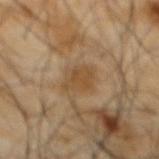<case>
<lighting>cross-polarized</lighting>
<image>
  <source>total-body photography crop</source>
  <field_of_view_mm>15</field_of_view_mm>
</image>
<patient>
  <sex>male</sex>
  <age_approx>65</age_approx>
</patient>
<site>mid back</site>
<automated_metrics>
  <nevus_likeness_0_100>25</nevus_likeness_0_100>
  <lesion_detection_confidence_0_100>100</lesion_detection_confidence_0_100>
</automated_metrics>
<lesion_size>
  <long_diameter_mm_approx>3.0</long_diameter_mm_approx>
</lesion_size>
</case>